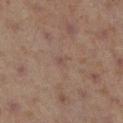The lesion was photographed on a routine skin check and not biopsied; there is no pathology result. This is a cross-polarized tile. The patient is a female approximately 40 years of age. Cropped from a whole-body photographic skin survey; the tile spans about 15 mm. The lesion is located on the right lower leg. The recorded lesion diameter is about 1.5 mm.